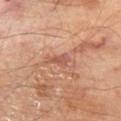Notes:
– follow-up: no biopsy performed (imaged during a skin exam)
– imaging modality: ~15 mm tile from a whole-body skin photo
– illumination: cross-polarized illumination
– subject: male, roughly 70 years of age
– anatomic site: the left thigh
– lesion diameter: about 2.5 mm
– automated metrics: a mean CIELAB color near L≈53 a*≈25 b*≈28; a border-irregularity rating of about 4/10, internal color variation of about 0.5 on a 0–10 scale, and a peripheral color-asymmetry measure near 0; a classifier nevus-likeness of about 0/100